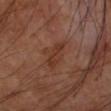Assessment: The lesion was tiled from a total-body skin photograph and was not biopsied. Context: The total-body-photography lesion software estimated a footprint of about 6.5 mm², a shape eccentricity near 0.85, and a shape-asymmetry score of about 0.25 (0 = symmetric). It also reported a border-irregularity index near 3/10, internal color variation of about 2.5 on a 0–10 scale, and peripheral color asymmetry of about 1. It also reported a detector confidence of about 100 out of 100 that the crop contains a lesion. On the left upper arm. Captured under cross-polarized illumination. Longest diameter approximately 4 mm. A male subject, aged around 70. A lesion tile, about 15 mm wide, cut from a 3D total-body photograph.